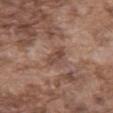Clinical impression: This lesion was catalogued during total-body skin photography and was not selected for biopsy. Acquisition and patient details: The lesion is on the mid back. Imaged with white-light lighting. A male subject approximately 75 years of age. A 15 mm crop from a total-body photograph taken for skin-cancer surveillance.